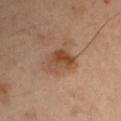biopsy status: no biopsy performed (imaged during a skin exam)
image: total-body-photography crop, ~15 mm field of view
image-analysis metrics: a lesion color around L≈45 a*≈21 b*≈31 in CIELAB, roughly 10 lightness units darker than nearby skin, and a normalized lesion–skin contrast near 8; a border-irregularity index near 3/10 and a color-variation rating of about 6.5/10; lesion-presence confidence of about 100/100
subject: male, approximately 50 years of age
diameter: ≈3.5 mm
site: the left upper arm